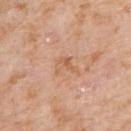Impression:
Part of a total-body skin-imaging series; this lesion was reviewed on a skin check and was not flagged for biopsy.
Context:
Captured under white-light illumination. A male patient, about 75 years old. A region of skin cropped from a whole-body photographic capture, roughly 15 mm wide. Located on the right upper arm. About 3 mm across.